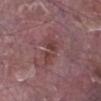Assessment: This lesion was catalogued during total-body skin photography and was not selected for biopsy. Background: A close-up tile cropped from a whole-body skin photograph, about 15 mm across. A male patient about 40 years old. Captured under white-light illumination. The lesion is located on the left lower leg. About 4.5 mm across. An algorithmic analysis of the crop reported a mean CIELAB color near L≈41 a*≈22 b*≈19 and a lesion-to-skin contrast of about 6 (normalized; higher = more distinct). It also reported a within-lesion color-variation index near 3.5/10 and peripheral color asymmetry of about 1. The analysis additionally found a classifier nevus-likeness of about 0/100 and a detector confidence of about 95 out of 100 that the crop contains a lesion.